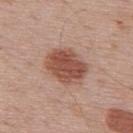<lesion>
<biopsy_status>not biopsied; imaged during a skin examination</biopsy_status>
<patient>
  <sex>male</sex>
  <age_approx>70</age_approx>
</patient>
<image>
  <source>total-body photography crop</source>
  <field_of_view_mm>15</field_of_view_mm>
</image>
<site>upper back</site>
</lesion>